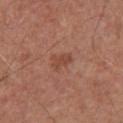The tile uses white-light illumination. A 15 mm close-up extracted from a 3D total-body photography capture. The lesion is located on the chest. A male subject, aged around 75. The recorded lesion diameter is about 3 mm.